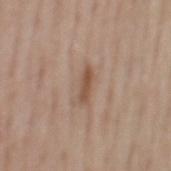imaging modality: total-body-photography crop, ~15 mm field of view | patient: male, in their mid- to late 50s | size: ~3.5 mm (longest diameter) | location: the mid back | lighting: white-light.